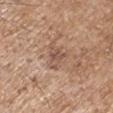Clinical impression:
The lesion was photographed on a routine skin check and not biopsied; there is no pathology result.
Acquisition and patient details:
The patient is a male in their 70s. On the right upper arm. Cropped from a whole-body photographic skin survey; the tile spans about 15 mm. Automated image analysis of the tile measured an area of roughly 6 mm², an eccentricity of roughly 0.75, and a symmetry-axis asymmetry near 0.5. It also reported a nevus-likeness score of about 0/100. Captured under white-light illumination.